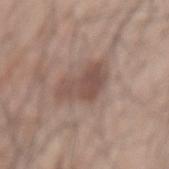Recorded during total-body skin imaging; not selected for excision or biopsy.
The patient is a male in their 70s.
This image is a 15 mm lesion crop taken from a total-body photograph.
Measured at roughly 5 mm in maximum diameter.
The lesion is on the mid back.
The lesion-visualizer software estimated a border-irregularity index near 4/10. The analysis additionally found a detector confidence of about 100 out of 100 that the crop contains a lesion.